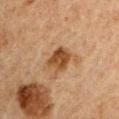Clinical impression: No biopsy was performed on this lesion — it was imaged during a full skin examination and was not determined to be concerning. Image and clinical context: A region of skin cropped from a whole-body photographic capture, roughly 15 mm wide. Captured under cross-polarized illumination. Located on the right upper arm. About 3 mm across. A male subject, about 50 years old.